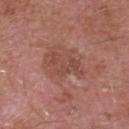workup = no biopsy performed (imaged during a skin exam); illumination = white-light; patient = male, aged 73 to 77; image source = 15 mm crop, total-body photography; TBP lesion metrics = a lesion color around L≈47 a*≈23 b*≈26 in CIELAB, roughly 7 lightness units darker than nearby skin, and a normalized border contrast of about 5.5; body site = the head or neck; lesion diameter = ≈4.5 mm.